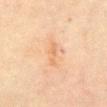Clinical impression: Recorded during total-body skin imaging; not selected for excision or biopsy. Acquisition and patient details: Imaged with cross-polarized lighting. The recorded lesion diameter is about 3.5 mm. The total-body-photography lesion software estimated a border-irregularity rating of about 5.5/10, internal color variation of about 0.5 on a 0–10 scale, and peripheral color asymmetry of about 0. The lesion is on the chest. A 15 mm crop from a total-body photograph taken for skin-cancer surveillance. A female patient, aged 68 to 72.